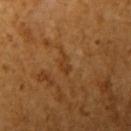Q: Is there a histopathology result?
A: total-body-photography surveillance lesion; no biopsy
Q: Lesion location?
A: the arm
Q: Automated lesion metrics?
A: a lesion color around L≈39 a*≈22 b*≈38 in CIELAB, a lesion–skin lightness drop of about 6, and a normalized border contrast of about 5.5; a color-variation rating of about 0/10 and radial color variation of about 0; a lesion-detection confidence of about 85/100
Q: How was the tile lit?
A: cross-polarized illumination
Q: What kind of image is this?
A: ~15 mm crop, total-body skin-cancer survey
Q: Patient demographics?
A: female, in their mid- to late 50s
Q: How large is the lesion?
A: ≈2.5 mm